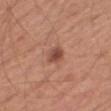The lesion was photographed on a routine skin check and not biopsied; there is no pathology result.
Measured at roughly 2.5 mm in maximum diameter.
From the leg.
A lesion tile, about 15 mm wide, cut from a 3D total-body photograph.
The patient is a male about 65 years old.